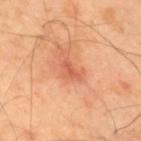Q: How was this image acquired?
A: ~15 mm crop, total-body skin-cancer survey
Q: Who is the patient?
A: male, roughly 40 years of age
Q: Where on the body is the lesion?
A: the back
Q: How large is the lesion?
A: ≈2.5 mm